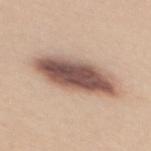The lesion was tiled from a total-body skin photograph and was not biopsied.
Located on the mid back.
The tile uses white-light illumination.
The patient is a female aged around 45.
Measured at roughly 8.5 mm in maximum diameter.
This image is a 15 mm lesion crop taken from a total-body photograph.